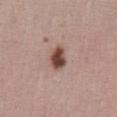Captured during whole-body skin photography for melanoma surveillance; the lesion was not biopsied. The total-body-photography lesion software estimated a lesion area of about 5.5 mm², an outline eccentricity of about 0.7 (0 = round, 1 = elongated), and two-axis asymmetry of about 0.2. The patient is a female about 50 years old. This is a white-light tile. Located on the abdomen. A lesion tile, about 15 mm wide, cut from a 3D total-body photograph.